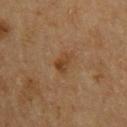The lesion was photographed on a routine skin check and not biopsied; there is no pathology result.
The patient is a male aged approximately 60.
An algorithmic analysis of the crop reported a lesion area of about 3.5 mm², a shape eccentricity near 0.8, and two-axis asymmetry of about 0.4. The analysis additionally found a border-irregularity rating of about 4/10. The software also gave a nevus-likeness score of about 40/100.
Cropped from a total-body skin-imaging series; the visible field is about 15 mm.
The lesion's longest dimension is about 2.5 mm.